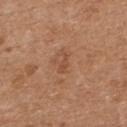Captured during whole-body skin photography for melanoma surveillance; the lesion was not biopsied.
Located on the back.
A male patient, aged around 70.
A roughly 15 mm field-of-view crop from a total-body skin photograph.